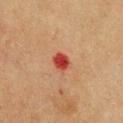The lesion was photographed on a routine skin check and not biopsied; there is no pathology result. A 15 mm close-up extracted from a 3D total-body photography capture. The lesion's longest dimension is about 2.5 mm. The patient is a female in their mid- to late 50s. The lesion is located on the chest.